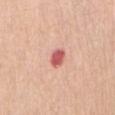The lesion was tiled from a total-body skin photograph and was not biopsied.